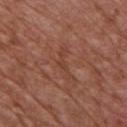Impression:
Part of a total-body skin-imaging series; this lesion was reviewed on a skin check and was not flagged for biopsy.
Context:
The lesion-visualizer software estimated a lesion color around L≈42 a*≈24 b*≈29 in CIELAB and roughly 5 lightness units darker than nearby skin. The software also gave a border-irregularity index near 9/10, internal color variation of about 0 on a 0–10 scale, and radial color variation of about 0. Located on the chest. The patient is a male in their mid- to late 70s. Imaged with white-light lighting. A lesion tile, about 15 mm wide, cut from a 3D total-body photograph.